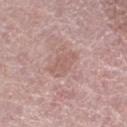Imaged during a routine full-body skin examination; the lesion was not biopsied and no histopathology is available. A female patient, aged 58 to 62. A close-up tile cropped from a whole-body skin photograph, about 15 mm across. From the left lower leg.